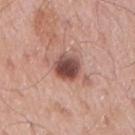Recorded during total-body skin imaging; not selected for excision or biopsy. From the right upper arm. The lesion's longest dimension is about 3 mm. A male subject aged 53–57. The tile uses white-light illumination. This image is a 15 mm lesion crop taken from a total-body photograph.